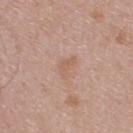body site=the upper back | image source=15 mm crop, total-body photography | TBP lesion metrics=two-axis asymmetry of about 0.45; a lesion color around L≈59 a*≈19 b*≈29 in CIELAB and a normalized lesion–skin contrast near 5; a color-variation rating of about 1/10 and radial color variation of about 0.5; a classifier nevus-likeness of about 0/100 and a lesion-detection confidence of about 100/100 | patient=male, aged 43–47 | lighting=white-light illumination.